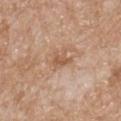This lesion was catalogued during total-body skin photography and was not selected for biopsy.
The subject is a female approximately 85 years of age or older.
Automated image analysis of the tile measured an average lesion color of about L≈56 a*≈20 b*≈33 (CIELAB), a lesion–skin lightness drop of about 9, and a normalized lesion–skin contrast near 6.5. The software also gave a nevus-likeness score of about 0/100 and lesion-presence confidence of about 100/100.
This is a white-light tile.
Cropped from a whole-body photographic skin survey; the tile spans about 15 mm.
The lesion is located on the upper back.
The recorded lesion diameter is about 2.5 mm.